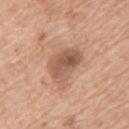Recorded during total-body skin imaging; not selected for excision or biopsy. The lesion is on the upper back. The subject is a female in their 40s. A roughly 15 mm field-of-view crop from a total-body skin photograph. Longest diameter approximately 4.5 mm. Imaged with white-light lighting.